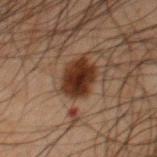notes = catalogued during a skin exam; not biopsied
automated lesion analysis = a lesion color around L≈26 a*≈18 b*≈24 in CIELAB and a normalized lesion–skin contrast near 13.5
lighting = cross-polarized illumination
size = ≈4.5 mm
site = the chest
patient = male, approximately 65 years of age
image = total-body-photography crop, ~15 mm field of view